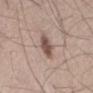Impression: This lesion was catalogued during total-body skin photography and was not selected for biopsy. Clinical summary: A male patient approximately 55 years of age. This is a white-light tile. Cropped from a whole-body photographic skin survey; the tile spans about 15 mm. About 3 mm across.